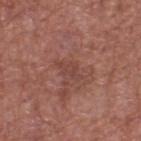biopsy status = imaged on a skin check; not biopsied
imaging modality = ~15 mm crop, total-body skin-cancer survey
patient = male, in their mid-70s
anatomic site = the back